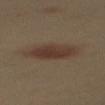  biopsy_status: not biopsied; imaged during a skin examination
  patient:
    sex: female
    age_approx: 40
  image:
    source: total-body photography crop
    field_of_view_mm: 15
  site: back